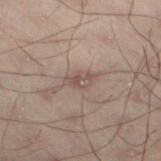Q: Was a biopsy performed?
A: imaged on a skin check; not biopsied
Q: How was this image acquired?
A: 15 mm crop, total-body photography
Q: What did automated image analysis measure?
A: a border-irregularity rating of about 2/10, a color-variation rating of about 3/10, and radial color variation of about 1
Q: Lesion location?
A: the left lower leg
Q: How was the tile lit?
A: cross-polarized illumination
Q: What are the patient's age and sex?
A: male, aged 48 to 52
Q: How large is the lesion?
A: about 3 mm